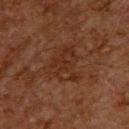follow-up: total-body-photography surveillance lesion; no biopsy | illumination: cross-polarized illumination | lesion diameter: ≈4.5 mm | location: the back | subject: male, about 60 years old | acquisition: ~15 mm crop, total-body skin-cancer survey.